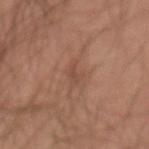Case summary:
* follow-up — no biopsy performed (imaged during a skin exam)
* patient — male, aged around 45
* anatomic site — the lower back
* lesion size — ~2.5 mm (longest diameter)
* automated lesion analysis — border irregularity of about 4.5 on a 0–10 scale, internal color variation of about 0 on a 0–10 scale, and a peripheral color-asymmetry measure near 0; a nevus-likeness score of about 0/100 and a lesion-detection confidence of about 95/100
* tile lighting — cross-polarized illumination
* acquisition — total-body-photography crop, ~15 mm field of view